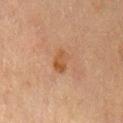Part of a total-body skin-imaging series; this lesion was reviewed on a skin check and was not flagged for biopsy. The lesion is located on the mid back. The tile uses cross-polarized illumination. A region of skin cropped from a whole-body photographic capture, roughly 15 mm wide. The total-body-photography lesion software estimated an area of roughly 5 mm², a shape eccentricity near 0.8, and two-axis asymmetry of about 0.2. The software also gave a lesion color around L≈43 a*≈19 b*≈31 in CIELAB, a lesion–skin lightness drop of about 7, and a normalized lesion–skin contrast near 6.5. And it measured a border-irregularity index near 2/10, a within-lesion color-variation index near 4/10, and radial color variation of about 1. It also reported a nevus-likeness score of about 10/100 and a detector confidence of about 100 out of 100 that the crop contains a lesion. The patient is a male aged around 70. The recorded lesion diameter is about 3 mm.